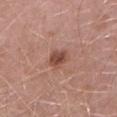Captured during whole-body skin photography for melanoma surveillance; the lesion was not biopsied. The recorded lesion diameter is about 2.5 mm. Imaged with white-light lighting. Cropped from a whole-body photographic skin survey; the tile spans about 15 mm. The patient is a male aged around 50. Located on the left lower leg. Automated tile analysis of the lesion measured an area of roughly 4.5 mm², an eccentricity of roughly 0.65, and two-axis asymmetry of about 0.25.